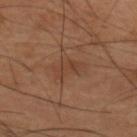Clinical impression: Imaged during a routine full-body skin examination; the lesion was not biopsied and no histopathology is available. Image and clinical context: The recorded lesion diameter is about 3.5 mm. The subject is a male aged approximately 60. Captured under cross-polarized illumination. Automated image analysis of the tile measured an outline eccentricity of about 0.8 (0 = round, 1 = elongated) and a shape-asymmetry score of about 0.35 (0 = symmetric). The analysis additionally found about 6 CIELAB-L* units darker than the surrounding skin and a normalized border contrast of about 5. The software also gave a border-irregularity rating of about 4/10, internal color variation of about 2 on a 0–10 scale, and peripheral color asymmetry of about 0.5. It also reported a nevus-likeness score of about 0/100 and a detector confidence of about 100 out of 100 that the crop contains a lesion. This image is a 15 mm lesion crop taken from a total-body photograph. The lesion is located on the upper back.